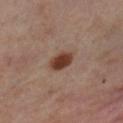diameter: ~3 mm (longest diameter)
image source: 15 mm crop, total-body photography
image-analysis metrics: an area of roughly 6 mm², an eccentricity of roughly 0.7, and a shape-asymmetry score of about 0.15 (0 = symmetric); a lesion color around L≈40 a*≈21 b*≈28 in CIELAB, roughly 14 lightness units darker than nearby skin, and a normalized border contrast of about 11.5; a lesion-detection confidence of about 100/100
patient: female, roughly 55 years of age
illumination: cross-polarized illumination
site: the left lower leg